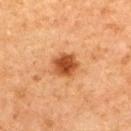Case summary:
* follow-up: imaged on a skin check; not biopsied